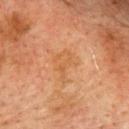Clinical impression: Captured during whole-body skin photography for melanoma surveillance; the lesion was not biopsied. Clinical summary: Located on the upper back. The tile uses cross-polarized illumination. Automated image analysis of the tile measured an automated nevus-likeness rating near 0 out of 100 and lesion-presence confidence of about 100/100. The subject is a male aged 73–77. A region of skin cropped from a whole-body photographic capture, roughly 15 mm wide. The lesion's longest dimension is about 4 mm.